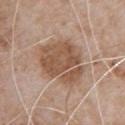• follow-up — no biopsy performed (imaged during a skin exam)
• patient — male, in their 80s
• body site — the chest
• acquisition — ~15 mm tile from a whole-body skin photo